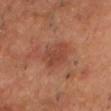Q: Was a biopsy performed?
A: catalogued during a skin exam; not biopsied
Q: What did automated image analysis measure?
A: a lesion area of about 9 mm², a shape eccentricity near 0.7, and a symmetry-axis asymmetry near 0.2; a border-irregularity index near 2/10 and internal color variation of about 2 on a 0–10 scale
Q: What kind of image is this?
A: ~15 mm tile from a whole-body skin photo
Q: What are the patient's age and sex?
A: male, about 65 years old
Q: Illumination type?
A: cross-polarized illumination
Q: What is the lesion's diameter?
A: ≈4 mm
Q: Lesion location?
A: the chest